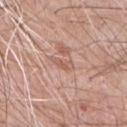  biopsy_status: not biopsied; imaged during a skin examination
  site: chest
  lesion_size:
    long_diameter_mm_approx: 3.0
  automated_metrics:
    cielab_L: 59
    cielab_a: 22
    cielab_b: 27
    vs_skin_darker_L: 8.0
    vs_skin_contrast_norm: 5.5
    border_irregularity_0_10: 4.0
    color_variation_0_10: 2.0
  image:
    source: total-body photography crop
    field_of_view_mm: 15
  patient:
    sex: male
    age_approx: 65